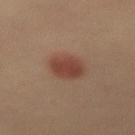biopsy status: total-body-photography surveillance lesion; no biopsy | lesion size: ~3.5 mm (longest diameter) | subject: female, aged around 50 | lighting: cross-polarized | image: ~15 mm tile from a whole-body skin photo.